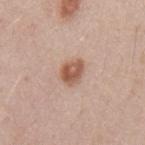{"biopsy_status": "not biopsied; imaged during a skin examination", "image": {"source": "total-body photography crop", "field_of_view_mm": 15}, "patient": {"sex": "male", "age_approx": 50}, "lighting": "white-light", "lesion_size": {"long_diameter_mm_approx": 3.0}, "automated_metrics": {"area_mm2_approx": 5.5, "eccentricity": 0.65, "shape_asymmetry": 0.25, "cielab_L": 56, "cielab_a": 22, "cielab_b": 29, "vs_skin_darker_L": 13.0, "vs_skin_contrast_norm": 9.0, "border_irregularity_0_10": 2.0, "color_variation_0_10": 4.5, "peripheral_color_asymmetry": 1.5, "nevus_likeness_0_100": 100, "lesion_detection_confidence_0_100": 100}, "site": "mid back"}